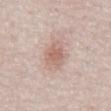This lesion was catalogued during total-body skin photography and was not selected for biopsy.
The lesion-visualizer software estimated a mean CIELAB color near L≈62 a*≈19 b*≈25, about 10 CIELAB-L* units darker than the surrounding skin, and a lesion-to-skin contrast of about 6.5 (normalized; higher = more distinct). It also reported a classifier nevus-likeness of about 70/100 and a lesion-detection confidence of about 100/100.
From the abdomen.
A female patient aged approximately 50.
About 4 mm across.
Cropped from a total-body skin-imaging series; the visible field is about 15 mm.
This is a white-light tile.